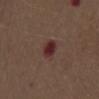workup=no biopsy performed (imaged during a skin exam) | size=≈3 mm | automated metrics=a border-irregularity rating of about 3/10, internal color variation of about 3.5 on a 0–10 scale, and peripheral color asymmetry of about 1.5 | subject=male, aged approximately 70 | imaging modality=~15 mm crop, total-body skin-cancer survey | lighting=white-light | anatomic site=the chest.